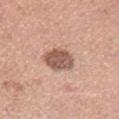Q: Was a biopsy performed?
A: imaged on a skin check; not biopsied
Q: How large is the lesion?
A: about 3.5 mm
Q: Automated lesion metrics?
A: a lesion area of about 9 mm², an outline eccentricity of about 0.55 (0 = round, 1 = elongated), and two-axis asymmetry of about 0.15; a border-irregularity index near 1.5/10
Q: Lesion location?
A: the left upper arm
Q: How was the tile lit?
A: white-light
Q: Who is the patient?
A: male, aged around 25
Q: What is the imaging modality?
A: ~15 mm tile from a whole-body skin photo